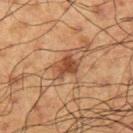Captured during whole-body skin photography for melanoma surveillance; the lesion was not biopsied.
About 3 mm across.
Located on the left upper arm.
A 15 mm close-up extracted from a 3D total-body photography capture.
Imaged with cross-polarized lighting.
The patient is a male in their 60s.
Automated image analysis of the tile measured a classifier nevus-likeness of about 75/100.